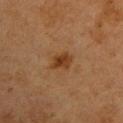The lesion was tiled from a total-body skin photograph and was not biopsied. Located on the left upper arm. Cropped from a whole-body photographic skin survey; the tile spans about 15 mm. A female patient, aged approximately 40.